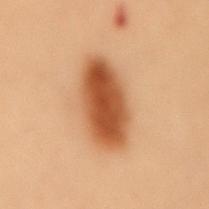Q: How large is the lesion?
A: about 7 mm
Q: What lighting was used for the tile?
A: cross-polarized
Q: Patient demographics?
A: female, aged 48–52
Q: What kind of image is this?
A: ~15 mm crop, total-body skin-cancer survey
Q: Lesion location?
A: the back